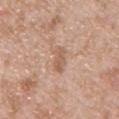The patient is a male roughly 50 years of age.
Cropped from a whole-body photographic skin survey; the tile spans about 15 mm.
This is a white-light tile.
Located on the front of the torso.